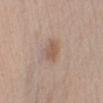{"biopsy_status": "not biopsied; imaged during a skin examination", "image": {"source": "total-body photography crop", "field_of_view_mm": 15}, "site": "right upper arm", "patient": {"sex": "male", "age_approx": 45}}